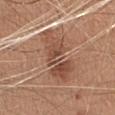{
  "biopsy_status": "not biopsied; imaged during a skin examination"
}